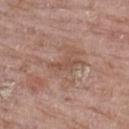{
  "biopsy_status": "not biopsied; imaged during a skin examination",
  "automated_metrics": {
    "area_mm2_approx": 4.5,
    "shape_asymmetry": 0.35,
    "cielab_L": 51,
    "cielab_a": 19,
    "cielab_b": 27,
    "vs_skin_darker_L": 7.0,
    "vs_skin_contrast_norm": 5.5,
    "border_irregularity_0_10": 5.0,
    "color_variation_0_10": 0.5,
    "nevus_likeness_0_100": 0
  },
  "site": "leg",
  "patient": {
    "sex": "female",
    "age_approx": "85+"
  },
  "lesion_size": {
    "long_diameter_mm_approx": 4.0
  },
  "lighting": "white-light",
  "image": {
    "source": "total-body photography crop",
    "field_of_view_mm": 15
  }
}